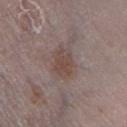Assessment: Recorded during total-body skin imaging; not selected for excision or biopsy.